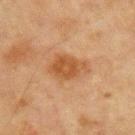Q: How large is the lesion?
A: ≈5 mm
Q: What is the anatomic site?
A: the chest
Q: Automated lesion metrics?
A: roughly 9 lightness units darker than nearby skin; border irregularity of about 3.5 on a 0–10 scale
Q: How was this image acquired?
A: 15 mm crop, total-body photography
Q: Patient demographics?
A: male, aged 63–67
Q: What lighting was used for the tile?
A: cross-polarized illumination